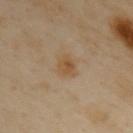notes — imaged on a skin check; not biopsied
location — the upper back
subject — female, in their 60s
lighting — cross-polarized
acquisition — 15 mm crop, total-body photography
diameter — about 2.5 mm